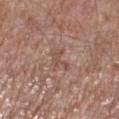Background: From the leg. A 15 mm crop from a total-body photograph taken for skin-cancer surveillance. A male subject approximately 55 years of age. The recorded lesion diameter is about 3 mm. Imaged with white-light lighting.